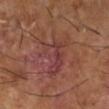follow-up: catalogued during a skin exam; not biopsied | body site: the leg | subject: male, about 65 years old | image source: total-body-photography crop, ~15 mm field of view.